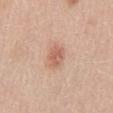body site=the abdomen; acquisition=15 mm crop, total-body photography; patient=male, approximately 65 years of age.